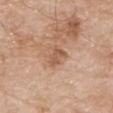Recorded during total-body skin imaging; not selected for excision or biopsy.
A 15 mm close-up extracted from a 3D total-body photography capture.
Approximately 3 mm at its widest.
The lesion is on the left upper arm.
The tile uses white-light illumination.
The patient is a male in their 80s.